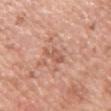notes: total-body-photography surveillance lesion; no biopsy | lighting: white-light illumination | lesion diameter: ~4 mm (longest diameter) | patient: female, aged 43 to 47 | body site: the right upper arm | automated lesion analysis: a lesion color around L≈58 a*≈23 b*≈30 in CIELAB and a normalized lesion–skin contrast near 5.5 | image: 15 mm crop, total-body photography.